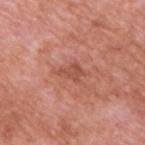| feature | finding |
|---|---|
| imaging modality | ~15 mm crop, total-body skin-cancer survey |
| patient | male, in their 60s |
| lesion diameter | ≈3 mm |
| body site | the upper back |